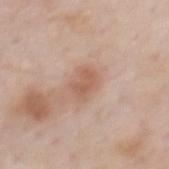biopsy status: imaged on a skin check; not biopsied
acquisition: total-body-photography crop, ~15 mm field of view
patient: male, aged 58–62
site: the mid back